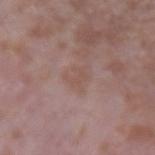Imaged during a routine full-body skin examination; the lesion was not biopsied and no histopathology is available. Imaged with white-light lighting. A 15 mm crop from a total-body photograph taken for skin-cancer surveillance. Measured at roughly 2.5 mm in maximum diameter. A male subject in their mid- to late 60s. An algorithmic analysis of the crop reported an area of roughly 3.5 mm², a shape eccentricity near 0.5, and a shape-asymmetry score of about 0.55 (0 = symmetric). The analysis additionally found a mean CIELAB color near L≈51 a*≈18 b*≈23, a lesion–skin lightness drop of about 5, and a lesion-to-skin contrast of about 4.5 (normalized; higher = more distinct). The analysis additionally found border irregularity of about 7 on a 0–10 scale, internal color variation of about 0 on a 0–10 scale, and radial color variation of about 0. And it measured an automated nevus-likeness rating near 0 out of 100 and lesion-presence confidence of about 100/100. On the left upper arm.